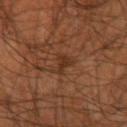The lesion was tiled from a total-body skin photograph and was not biopsied.
Located on the right upper arm.
This is a cross-polarized tile.
The lesion-visualizer software estimated a shape eccentricity near 0.8 and a symmetry-axis asymmetry near 0.5.
The patient is a male roughly 45 years of age.
A lesion tile, about 15 mm wide, cut from a 3D total-body photograph.
About 3 mm across.